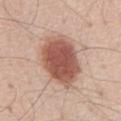This is a white-light tile. An algorithmic analysis of the crop reported an eccentricity of roughly 0.75 and a shape-asymmetry score of about 0.15 (0 = symmetric). It also reported an average lesion color of about L≈55 a*≈23 b*≈27 (CIELAB), a lesion–skin lightness drop of about 16, and a lesion-to-skin contrast of about 10.5 (normalized; higher = more distinct). And it measured border irregularity of about 1.5 on a 0–10 scale, internal color variation of about 4.5 on a 0–10 scale, and radial color variation of about 1. The lesion is located on the abdomen. The lesion's longest dimension is about 7 mm. A male patient aged around 70. A region of skin cropped from a whole-body photographic capture, roughly 15 mm wide.